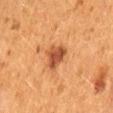Q: Was this lesion biopsied?
A: catalogued during a skin exam; not biopsied
Q: Patient demographics?
A: female, approximately 50 years of age
Q: What did automated image analysis measure?
A: an average lesion color of about L≈42 a*≈25 b*≈33 (CIELAB), a lesion–skin lightness drop of about 12, and a normalized lesion–skin contrast near 9.5; a border-irregularity index near 2.5/10, a within-lesion color-variation index near 2.5/10, and radial color variation of about 1; an automated nevus-likeness rating near 85 out of 100 and a detector confidence of about 100 out of 100 that the crop contains a lesion
Q: What kind of image is this?
A: ~15 mm crop, total-body skin-cancer survey
Q: Where on the body is the lesion?
A: the lower back
Q: Illumination type?
A: cross-polarized
Q: What is the lesion's diameter?
A: about 3 mm